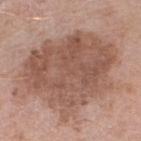Impression:
The lesion was photographed on a routine skin check and not biopsied; there is no pathology result.
Acquisition and patient details:
Automated image analysis of the tile measured an average lesion color of about L≈53 a*≈20 b*≈26 (CIELAB), about 11 CIELAB-L* units darker than the surrounding skin, and a normalized lesion–skin contrast near 7.5. The analysis additionally found a border-irregularity index near 3.5/10 and internal color variation of about 4.5 on a 0–10 scale. A 15 mm crop from a total-body photograph taken for skin-cancer surveillance. A female subject, aged approximately 70. The lesion is located on the left lower leg. Captured under white-light illumination. The recorded lesion diameter is about 10.5 mm.